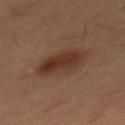{"biopsy_status": "not biopsied; imaged during a skin examination", "lighting": "cross-polarized", "automated_metrics": {"cielab_L": 29, "cielab_a": 17, "cielab_b": 23, "vs_skin_darker_L": 8.0, "vs_skin_contrast_norm": 8.0, "border_irregularity_0_10": 2.0, "color_variation_0_10": 4.0, "peripheral_color_asymmetry": 1.5}, "patient": {"sex": "male", "age_approx": 55}, "lesion_size": {"long_diameter_mm_approx": 5.5}, "site": "abdomen", "image": {"source": "total-body photography crop", "field_of_view_mm": 15}}